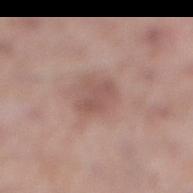biopsy status: catalogued during a skin exam; not biopsied | tile lighting: white-light | imaging modality: 15 mm crop, total-body photography | subject: male, roughly 55 years of age | image-analysis metrics: an outline eccentricity of about 0.8 (0 = round, 1 = elongated) and a symmetry-axis asymmetry near 0.4; a lesion color around L≈53 a*≈20 b*≈23 in CIELAB, about 8 CIELAB-L* units darker than the surrounding skin, and a normalized lesion–skin contrast near 5.5; border irregularity of about 4.5 on a 0–10 scale, internal color variation of about 1.5 on a 0–10 scale, and peripheral color asymmetry of about 0.5; a nevus-likeness score of about 5/100 and lesion-presence confidence of about 100/100 | body site: the right lower leg.